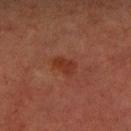Findings:
* notes: catalogued during a skin exam; not biopsied
* body site: the left lower leg
* patient: male, in their 70s
* lighting: cross-polarized
* diameter: about 3 mm
* imaging modality: 15 mm crop, total-body photography
* TBP lesion metrics: a footprint of about 5 mm² and a shape eccentricity near 0.75; a border-irregularity index near 2.5/10 and peripheral color asymmetry of about 0.5; an automated nevus-likeness rating near 5 out of 100 and a lesion-detection confidence of about 100/100